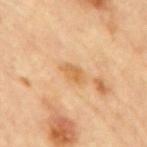workup=imaged on a skin check; not biopsied | subject=male, aged approximately 85 or older | image=~15 mm tile from a whole-body skin photo | site=the mid back.